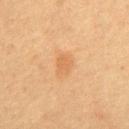| feature | finding |
|---|---|
| site | the chest |
| imaging modality | ~15 mm crop, total-body skin-cancer survey |
| diameter | about 3 mm |
| subject | male, approximately 60 years of age |
| tile lighting | cross-polarized illumination |